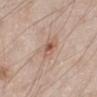The lesion was photographed on a routine skin check and not biopsied; there is no pathology result. This image is a 15 mm lesion crop taken from a total-body photograph. Captured under white-light illumination. From the leg. The patient is a male aged 58–62.